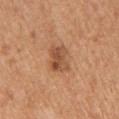{
  "biopsy_status": "not biopsied; imaged during a skin examination",
  "image": {
    "source": "total-body photography crop",
    "field_of_view_mm": 15
  },
  "lighting": "white-light",
  "patient": {
    "sex": "female",
    "age_approx": 55
  },
  "site": "arm",
  "lesion_size": {
    "long_diameter_mm_approx": 3.5
  }
}